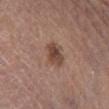Captured under white-light illumination. Located on the right lower leg. A lesion tile, about 15 mm wide, cut from a 3D total-body photograph. A male subject, in their mid- to late 60s. Approximately 3 mm at its widest.